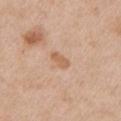Clinical impression:
Part of a total-body skin-imaging series; this lesion was reviewed on a skin check and was not flagged for biopsy.
Clinical summary:
The patient is a male approximately 65 years of age. Approximately 2.5 mm at its widest. The tile uses white-light illumination. A roughly 15 mm field-of-view crop from a total-body skin photograph. The lesion is on the left upper arm.